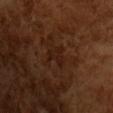Assessment: Part of a total-body skin-imaging series; this lesion was reviewed on a skin check and was not flagged for biopsy. Image and clinical context: Longest diameter approximately 2.5 mm. The patient is a male aged 63 to 67. Imaged with cross-polarized lighting. A 15 mm crop from a total-body photograph taken for skin-cancer surveillance.